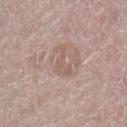Captured during whole-body skin photography for melanoma surveillance; the lesion was not biopsied. The tile uses white-light illumination. A lesion tile, about 15 mm wide, cut from a 3D total-body photograph. A female subject aged 38–42. Automated image analysis of the tile measured border irregularity of about 7 on a 0–10 scale, internal color variation of about 1 on a 0–10 scale, and a peripheral color-asymmetry measure near 0.5. Located on the right lower leg. Approximately 3.5 mm at its widest.